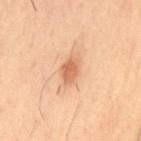follow-up — imaged on a skin check; not biopsied | automated lesion analysis — an area of roughly 5.5 mm², an outline eccentricity of about 0.8 (0 = round, 1 = elongated), and two-axis asymmetry of about 0.2; an average lesion color of about L≈64 a*≈24 b*≈37 (CIELAB), roughly 11 lightness units darker than nearby skin, and a normalized lesion–skin contrast near 7; border irregularity of about 2 on a 0–10 scale and internal color variation of about 2.5 on a 0–10 scale | imaging modality — 15 mm crop, total-body photography | tile lighting — cross-polarized illumination | anatomic site — the back | patient — male, aged around 40.